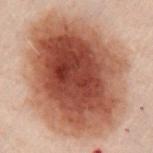No biopsy was performed on this lesion — it was imaged during a full skin examination and was not determined to be concerning. A 15 mm close-up extracted from a 3D total-body photography capture. This is a cross-polarized tile. Measured at roughly 12 mm in maximum diameter. On the chest. The patient is a male in their 50s. Automated tile analysis of the lesion measured a lesion area of about 100 mm² and a shape-asymmetry score of about 0.1 (0 = symmetric). It also reported a mean CIELAB color near L≈42 a*≈20 b*≈26, about 16 CIELAB-L* units darker than the surrounding skin, and a lesion-to-skin contrast of about 12 (normalized; higher = more distinct). The software also gave a nevus-likeness score of about 100/100 and a detector confidence of about 100 out of 100 that the crop contains a lesion.